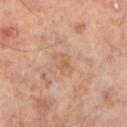Part of a total-body skin-imaging series; this lesion was reviewed on a skin check and was not flagged for biopsy. On the left lower leg. Automated tile analysis of the lesion measured a border-irregularity rating of about 2/10, internal color variation of about 3.5 on a 0–10 scale, and radial color variation of about 1. The analysis additionally found a lesion-detection confidence of about 100/100. Cropped from a whole-body photographic skin survey; the tile spans about 15 mm. The patient is a male approximately 50 years of age. The tile uses cross-polarized illumination. Measured at roughly 3 mm in maximum diameter.